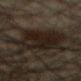| key | value |
|---|---|
| workup | no biopsy performed (imaged during a skin exam) |
| subject | male, aged 63–67 |
| acquisition | ~15 mm crop, total-body skin-cancer survey |
| body site | the chest |